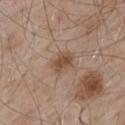biopsy_status: not biopsied; imaged during a skin examination
lesion_size:
  long_diameter_mm_approx: 3.0
image:
  source: total-body photography crop
  field_of_view_mm: 15
automated_metrics:
  area_mm2_approx: 5.5
  eccentricity: 0.7
  shape_asymmetry: 0.2
  color_variation_0_10: 3.0
  peripheral_color_asymmetry: 1.0
  lesion_detection_confidence_0_100: 100
site: back
patient:
  sex: male
  age_approx: 55
lighting: white-light